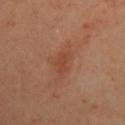Imaged during a routine full-body skin examination; the lesion was not biopsied and no histopathology is available. A male patient aged around 40. From the chest. A 15 mm crop from a total-body photograph taken for skin-cancer surveillance. The lesion's longest dimension is about 2.5 mm. Captured under cross-polarized illumination.